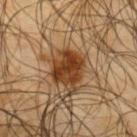Impression:
No biopsy was performed on this lesion — it was imaged during a full skin examination and was not determined to be concerning.
Acquisition and patient details:
A 15 mm close-up extracted from a 3D total-body photography capture. The recorded lesion diameter is about 4 mm. A male subject, aged around 65. The lesion is on the upper back. Imaged with cross-polarized lighting.